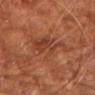workup — total-body-photography surveillance lesion; no biopsy
tile lighting — cross-polarized
patient — male, aged approximately 65
image — total-body-photography crop, ~15 mm field of view
body site — the left upper arm
size — ≈7.5 mm